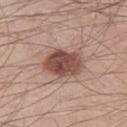notes: imaged on a skin check; not biopsied
image source: ~15 mm crop, total-body skin-cancer survey
site: the left thigh
subject: male, aged approximately 30